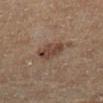biopsy_status: not biopsied; imaged during a skin examination
lesion_size:
  long_diameter_mm_approx: 3.0
patient:
  sex: female
  age_approx: 65
site: left lower leg
image:
  source: total-body photography crop
  field_of_view_mm: 15
lighting: cross-polarized